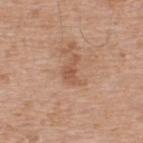The lesion was photographed on a routine skin check and not biopsied; there is no pathology result. From the upper back. The subject is a male in their mid- to late 50s. A roughly 15 mm field-of-view crop from a total-body skin photograph.